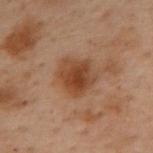Clinical impression:
This lesion was catalogued during total-body skin photography and was not selected for biopsy.
Background:
Longest diameter approximately 4 mm. This is a cross-polarized tile. This image is a 15 mm lesion crop taken from a total-body photograph. From the upper back. The patient is a female in their mid-50s. Automated image analysis of the tile measured a footprint of about 11 mm². It also reported a mean CIELAB color near L≈36 a*≈19 b*≈28 and a lesion–skin lightness drop of about 9. The software also gave an automated nevus-likeness rating near 90 out of 100 and lesion-presence confidence of about 100/100.